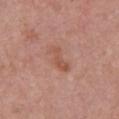Impression:
Captured during whole-body skin photography for melanoma surveillance; the lesion was not biopsied.